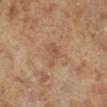  biopsy_status: not biopsied; imaged during a skin examination
  site: right lower leg
  image:
    source: total-body photography crop
    field_of_view_mm: 15
  patient:
    sex: female
    age_approx: 70